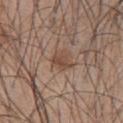notes: total-body-photography surveillance lesion; no biopsy | subject: male, approximately 45 years of age | image: ~15 mm crop, total-body skin-cancer survey | location: the front of the torso.